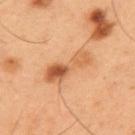* biopsy status — no biopsy performed (imaged during a skin exam)